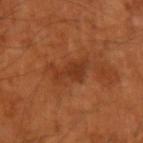follow-up = total-body-photography surveillance lesion; no biopsy | patient = male, aged 48 to 52 | body site = the left upper arm | image source = 15 mm crop, total-body photography | lighting = cross-polarized.